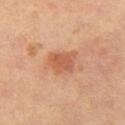{
  "biopsy_status": "not biopsied; imaged during a skin examination",
  "site": "left thigh",
  "lighting": "cross-polarized",
  "image": {
    "source": "total-body photography crop",
    "field_of_view_mm": 15
  },
  "lesion_size": {
    "long_diameter_mm_approx": 3.0
  },
  "patient": {
    "sex": "male",
    "age_approx": 65
  }
}